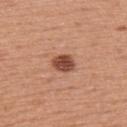Clinical impression:
No biopsy was performed on this lesion — it was imaged during a full skin examination and was not determined to be concerning.
Acquisition and patient details:
Cropped from a total-body skin-imaging series; the visible field is about 15 mm. The tile uses white-light illumination. A male subject, approximately 55 years of age. Longest diameter approximately 3 mm. The lesion is located on the upper back.